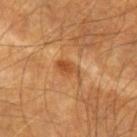workup: catalogued during a skin exam; not biopsied | subject: male, approximately 65 years of age | acquisition: 15 mm crop, total-body photography | location: the right forearm | illumination: cross-polarized illumination | size: ~3.5 mm (longest diameter).